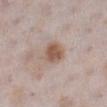Cropped from a whole-body photographic skin survey; the tile spans about 15 mm.
The lesion-visualizer software estimated a normalized lesion–skin contrast near 8.5. It also reported border irregularity of about 1 on a 0–10 scale, internal color variation of about 3.5 on a 0–10 scale, and radial color variation of about 1. It also reported a detector confidence of about 100 out of 100 that the crop contains a lesion.
The recorded lesion diameter is about 3 mm.
A female patient, aged around 30.
On the left lower leg.